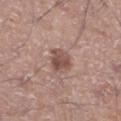The lesion was photographed on a routine skin check and not biopsied; there is no pathology result. Located on the left lower leg. A male subject, aged 53 to 57. Automated tile analysis of the lesion measured an area of roughly 6 mm² and a symmetry-axis asymmetry near 0.3. The software also gave a color-variation rating of about 4/10 and peripheral color asymmetry of about 1.5. It also reported an automated nevus-likeness rating near 75 out of 100 and a detector confidence of about 100 out of 100 that the crop contains a lesion. The lesion's longest dimension is about 3 mm. This image is a 15 mm lesion crop taken from a total-body photograph.